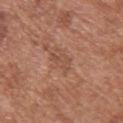Notes:
• workup · no biopsy performed (imaged during a skin exam)
• acquisition · ~15 mm tile from a whole-body skin photo
• site · the upper back
• subject · male, about 75 years old